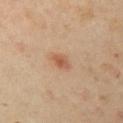{
  "biopsy_status": "not biopsied; imaged during a skin examination",
  "site": "left upper arm",
  "patient": {
    "sex": "female",
    "age_approx": 40
  },
  "image": {
    "source": "total-body photography crop",
    "field_of_view_mm": 15
  }
}